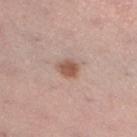Captured during whole-body skin photography for melanoma surveillance; the lesion was not biopsied. The recorded lesion diameter is about 2.5 mm. On the left lower leg. A lesion tile, about 15 mm wide, cut from a 3D total-body photograph. The patient is a female in their mid- to late 30s. The total-body-photography lesion software estimated a footprint of about 4.5 mm², an outline eccentricity of about 0.6 (0 = round, 1 = elongated), and a symmetry-axis asymmetry near 0.25. The analysis additionally found an average lesion color of about L≈55 a*≈20 b*≈27 (CIELAB), a lesion–skin lightness drop of about 11, and a normalized lesion–skin contrast near 8.5. It also reported internal color variation of about 2 on a 0–10 scale and a peripheral color-asymmetry measure near 0.5. It also reported a nevus-likeness score of about 95/100 and a lesion-detection confidence of about 100/100.